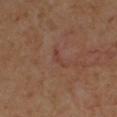This lesion was catalogued during total-body skin photography and was not selected for biopsy.
This is a cross-polarized tile.
From the left lower leg.
The subject is a female in their 50s.
Approximately 2.5 mm at its widest.
Cropped from a total-body skin-imaging series; the visible field is about 15 mm.